The lesion is located on the chest; approximately 7.5 mm at its widest; the patient is a male in their 60s; this image is a 15 mm lesion crop taken from a total-body photograph; this is a cross-polarized tile; The lesion-visualizer software estimated a footprint of about 15 mm², a shape eccentricity near 0.9, and two-axis asymmetry of about 0.5. The analysis additionally found a lesion color around L≈34 a*≈21 b*≈24 in CIELAB, a lesion–skin lightness drop of about 7, and a normalized border contrast of about 7. The software also gave an automated nevus-likeness rating near 0 out of 100 and a lesion-detection confidence of about 5/100.
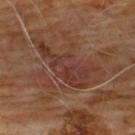diagnosis = a nodular basal cell carcinoma.